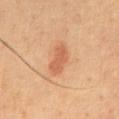Captured during whole-body skin photography for melanoma surveillance; the lesion was not biopsied.
The subject is a male in their mid-60s.
A close-up tile cropped from a whole-body skin photograph, about 15 mm across.
This is a cross-polarized tile.
Approximately 4.5 mm at its widest.
Located on the chest.